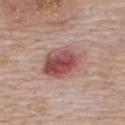No biopsy was performed on this lesion — it was imaged during a full skin examination and was not determined to be concerning.
Automated image analysis of the tile measured a lesion color around L≈51 a*≈25 b*≈23 in CIELAB, about 14 CIELAB-L* units darker than the surrounding skin, and a lesion-to-skin contrast of about 9.5 (normalized; higher = more distinct).
A male patient aged 73–77.
A 15 mm close-up extracted from a 3D total-body photography capture.
Measured at roughly 6 mm in maximum diameter.
The lesion is on the upper back.
This is a white-light tile.